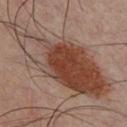<tbp_lesion>
<biopsy_status>not biopsied; imaged during a skin examination</biopsy_status>
<image>
  <source>total-body photography crop</source>
  <field_of_view_mm>15</field_of_view_mm>
</image>
<lighting>cross-polarized</lighting>
<site>chest</site>
<patient>
  <sex>male</sex>
  <age_approx>35</age_approx>
</patient>
<lesion_size>
  <long_diameter_mm_approx>14.5</long_diameter_mm_approx>
</lesion_size>
</tbp_lesion>